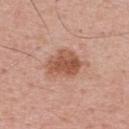<record>
  <biopsy_status>not biopsied; imaged during a skin examination</biopsy_status>
  <lighting>white-light</lighting>
  <lesion_size>
    <long_diameter_mm_approx>4.5</long_diameter_mm_approx>
  </lesion_size>
  <patient>
    <sex>male</sex>
    <age_approx>65</age_approx>
  </patient>
  <image>
    <source>total-body photography crop</source>
    <field_of_view_mm>15</field_of_view_mm>
  </image>
  <site>upper back</site>
</record>